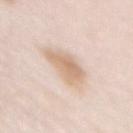notes: no biopsy performed (imaged during a skin exam) | patient: female, in their mid- to late 60s | image source: ~15 mm tile from a whole-body skin photo | diameter: ≈4.5 mm | anatomic site: the chest.